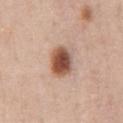Recorded during total-body skin imaging; not selected for excision or biopsy.
This is a white-light tile.
The total-body-photography lesion software estimated a symmetry-axis asymmetry near 0.05. And it measured an automated nevus-likeness rating near 100 out of 100.
On the chest.
A 15 mm close-up tile from a total-body photography series done for melanoma screening.
A male subject roughly 50 years of age.
Approximately 4 mm at its widest.